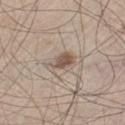The lesion was photographed on a routine skin check and not biopsied; there is no pathology result. Imaged with white-light lighting. On the leg. The total-body-photography lesion software estimated an area of roughly 6.5 mm², a shape eccentricity near 0.75, and two-axis asymmetry of about 0.35. The software also gave a mean CIELAB color near L≈55 a*≈13 b*≈25, about 11 CIELAB-L* units darker than the surrounding skin, and a normalized border contrast of about 7.5. The analysis additionally found internal color variation of about 3.5 on a 0–10 scale and peripheral color asymmetry of about 1. A male subject, in their mid- to late 40s. About 3.5 mm across. Cropped from a total-body skin-imaging series; the visible field is about 15 mm.